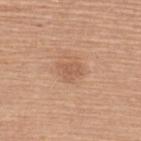Recorded during total-body skin imaging; not selected for excision or biopsy. The recorded lesion diameter is about 2.5 mm. A lesion tile, about 15 mm wide, cut from a 3D total-body photograph. An algorithmic analysis of the crop reported an automated nevus-likeness rating near 15 out of 100 and a lesion-detection confidence of about 100/100. The patient is a female aged 58–62. Located on the upper back. The tile uses white-light illumination.